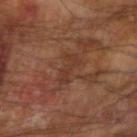{
  "biopsy_status": "not biopsied; imaged during a skin examination",
  "site": "left arm",
  "image": {
    "source": "total-body photography crop",
    "field_of_view_mm": 15
  },
  "patient": {
    "sex": "male",
    "age_approx": 65
  }
}